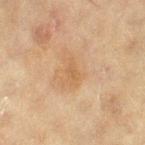Captured during whole-body skin photography for melanoma surveillance; the lesion was not biopsied. A female subject, aged approximately 80. Longest diameter approximately 2.5 mm. Automated image analysis of the tile measured a lesion area of about 2 mm², a shape eccentricity near 0.9, and two-axis asymmetry of about 0.45. The analysis additionally found a nevus-likeness score of about 0/100 and a detector confidence of about 100 out of 100 that the crop contains a lesion. The lesion is located on the right thigh. A 15 mm crop from a total-body photograph taken for skin-cancer surveillance.